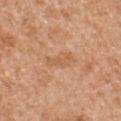notes: total-body-photography surveillance lesion; no biopsy | subject: male, about 55 years old | size: about 3.5 mm | image source: total-body-photography crop, ~15 mm field of view | illumination: white-light illumination | location: the chest | automated lesion analysis: a footprint of about 4.5 mm², an eccentricity of roughly 0.9, and a symmetry-axis asymmetry near 0.3; an average lesion color of about L≈59 a*≈23 b*≈37 (CIELAB) and a normalized lesion–skin contrast near 4.5.